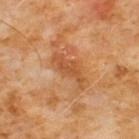Q: Lesion size?
A: ~5 mm (longest diameter)
Q: Illumination type?
A: cross-polarized
Q: What are the patient's age and sex?
A: male, aged 58–62
Q: How was this image acquired?
A: ~15 mm crop, total-body skin-cancer survey
Q: Where on the body is the lesion?
A: the chest
Q: Automated lesion metrics?
A: an area of roughly 8 mm², an eccentricity of roughly 0.9, and two-axis asymmetry of about 0.35; a border-irregularity rating of about 4.5/10, internal color variation of about 2 on a 0–10 scale, and radial color variation of about 0.5